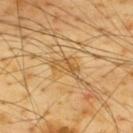Captured during whole-body skin photography for melanoma surveillance; the lesion was not biopsied. Automated image analysis of the tile measured an average lesion color of about L≈59 a*≈18 b*≈44 (CIELAB), about 8 CIELAB-L* units darker than the surrounding skin, and a normalized border contrast of about 6. It also reported border irregularity of about 5 on a 0–10 scale, a color-variation rating of about 4/10, and radial color variation of about 1.5. And it measured a detector confidence of about 80 out of 100 that the crop contains a lesion. A male patient, aged 63 to 67. A 15 mm close-up extracted from a 3D total-body photography capture. The lesion's longest dimension is about 3.5 mm. The lesion is located on the upper back.